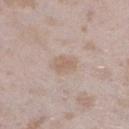| key | value |
|---|---|
| biopsy status | total-body-photography surveillance lesion; no biopsy |
| image | 15 mm crop, total-body photography |
| lesion diameter | about 3 mm |
| anatomic site | the left lower leg |
| patient | female, aged 23 to 27 |
| image-analysis metrics | internal color variation of about 1.5 on a 0–10 scale; a classifier nevus-likeness of about 5/100 and lesion-presence confidence of about 100/100 |
| lighting | white-light |